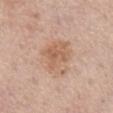| key | value |
|---|---|
| workup | catalogued during a skin exam; not biopsied |